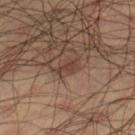{
  "biopsy_status": "not biopsied; imaged during a skin examination",
  "automated_metrics": {
    "area_mm2_approx": 2.5,
    "eccentricity": 0.9,
    "shape_asymmetry": 0.45,
    "cielab_L": 35,
    "cielab_a": 17,
    "cielab_b": 22,
    "vs_skin_darker_L": 7.0,
    "vs_skin_contrast_norm": 6.5,
    "color_variation_0_10": 0.5,
    "peripheral_color_asymmetry": 0.0,
    "nevus_likeness_0_100": 5
  },
  "image": {
    "source": "total-body photography crop",
    "field_of_view_mm": 15
  },
  "lighting": "cross-polarized",
  "patient": {
    "sex": "male",
    "age_approx": 35
  },
  "lesion_size": {
    "long_diameter_mm_approx": 2.5
  },
  "site": "right lower leg"
}